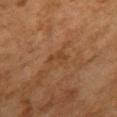Impression:
The lesion was tiled from a total-body skin photograph and was not biopsied.
Context:
A female patient, approximately 60 years of age. Approximately 2.5 mm at its widest. Automated image analysis of the tile measured a lesion area of about 3 mm², an eccentricity of roughly 0.85, and two-axis asymmetry of about 0.45. It also reported a lesion color around L≈36 a*≈19 b*≈31 in CIELAB, a lesion–skin lightness drop of about 5, and a normalized border contrast of about 5. A 15 mm close-up extracted from a 3D total-body photography capture. The tile uses cross-polarized illumination. The lesion is located on the front of the torso.